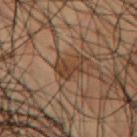| feature | finding |
|---|---|
| subject | male, aged 53 to 57 |
| automated metrics | an area of roughly 5.5 mm², an eccentricity of roughly 0.75, and a symmetry-axis asymmetry near 0.55; a border-irregularity rating of about 7/10 and a color-variation rating of about 3/10; a classifier nevus-likeness of about 10/100 |
| diameter | ~3.5 mm (longest diameter) |
| image | ~15 mm tile from a whole-body skin photo |
| body site | the chest |